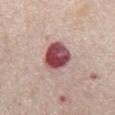| key | value |
|---|---|
| biopsy status | catalogued during a skin exam; not biopsied |
| diameter | ~4.5 mm (longest diameter) |
| patient | male, approximately 75 years of age |
| image | 15 mm crop, total-body photography |
| anatomic site | the chest |